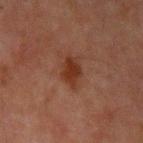Assessment:
The lesion was photographed on a routine skin check and not biopsied; there is no pathology result.
Clinical summary:
The total-body-photography lesion software estimated border irregularity of about 3 on a 0–10 scale and a within-lesion color-variation index near 2/10. The software also gave a classifier nevus-likeness of about 75/100 and lesion-presence confidence of about 100/100. The patient is a male in their 60s. Captured under cross-polarized illumination. A 15 mm crop from a total-body photograph taken for skin-cancer surveillance. On the arm.